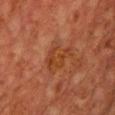Assessment:
The lesion was photographed on a routine skin check and not biopsied; there is no pathology result.
Clinical summary:
A lesion tile, about 15 mm wide, cut from a 3D total-body photograph. Automated tile analysis of the lesion measured a mean CIELAB color near L≈34 a*≈23 b*≈32, roughly 5 lightness units darker than nearby skin, and a lesion-to-skin contrast of about 6 (normalized; higher = more distinct). It also reported a nevus-likeness score of about 0/100 and a detector confidence of about 100 out of 100 that the crop contains a lesion. The lesion is located on the chest. A male patient aged 58–62. Longest diameter approximately 4 mm. The tile uses cross-polarized illumination.